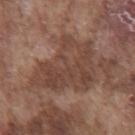{
  "image": {
    "source": "total-body photography crop",
    "field_of_view_mm": 15
  },
  "lesion_size": {
    "long_diameter_mm_approx": 7.5
  },
  "patient": {
    "sex": "male",
    "age_approx": 75
  },
  "lighting": "white-light",
  "site": "chest"
}